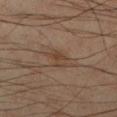Context: This image is a 15 mm lesion crop taken from a total-body photograph. A male subject roughly 45 years of age. Automated image analysis of the tile measured a color-variation rating of about 3/10 and radial color variation of about 1. The lesion is on the right lower leg. Approximately 3 mm at its widest.